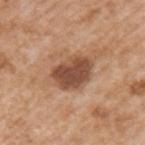Part of a total-body skin-imaging series; this lesion was reviewed on a skin check and was not flagged for biopsy.
A male subject, in their mid-60s.
Measured at roughly 4.5 mm in maximum diameter.
From the left upper arm.
A 15 mm crop from a total-body photograph taken for skin-cancer surveillance.